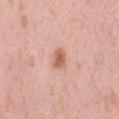Captured during whole-body skin photography for melanoma surveillance; the lesion was not biopsied.
The lesion's longest dimension is about 2.5 mm.
The lesion is located on the left thigh.
A roughly 15 mm field-of-view crop from a total-body skin photograph.
The lesion-visualizer software estimated a footprint of about 4 mm² and an eccentricity of roughly 0.7.
The patient is a female approximately 40 years of age.